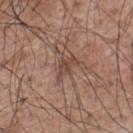The lesion was tiled from a total-body skin photograph and was not biopsied. A roughly 15 mm field-of-view crop from a total-body skin photograph. A male subject, approximately 70 years of age. Approximately 3 mm at its widest. The lesion is on the upper back. Automated tile analysis of the lesion measured a footprint of about 4.5 mm² and a symmetry-axis asymmetry near 0.4. It also reported a mean CIELAB color near L≈46 a*≈19 b*≈25 and a normalized border contrast of about 6.5.